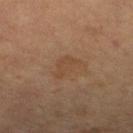Acquisition and patient details: Longest diameter approximately 3.5 mm. A female subject, aged 68 to 72. This is a cross-polarized tile. The lesion is on the right lower leg. A lesion tile, about 15 mm wide, cut from a 3D total-body photograph.